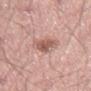• workup: total-body-photography surveillance lesion; no biopsy
• image: ~15 mm tile from a whole-body skin photo
• patient: male, approximately 55 years of age
• lesion diameter: ≈3.5 mm
• body site: the right thigh